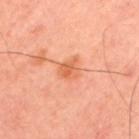Captured during whole-body skin photography for melanoma surveillance; the lesion was not biopsied.
A region of skin cropped from a whole-body photographic capture, roughly 15 mm wide.
A male subject, aged approximately 50.
Approximately 3 mm at its widest.
The lesion is on the back.